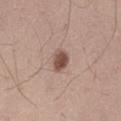Assessment:
The lesion was tiled from a total-body skin photograph and was not biopsied.
Image and clinical context:
The lesion is located on the left thigh. The tile uses white-light illumination. This image is a 15 mm lesion crop taken from a total-body photograph. The patient is a male aged around 45. An algorithmic analysis of the crop reported a color-variation rating of about 3.5/10.